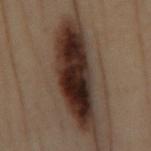Imaged during a routine full-body skin examination; the lesion was not biopsied and no histopathology is available. A female subject, approximately 50 years of age. The lesion is on the right lower leg. Longest diameter approximately 12 mm. This image is a 15 mm lesion crop taken from a total-body photograph.